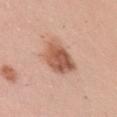biopsy status = total-body-photography surveillance lesion; no biopsy | imaging modality = ~15 mm crop, total-body skin-cancer survey | subject = female, roughly 40 years of age | size = ~4.5 mm (longest diameter) | body site = the right upper arm | automated metrics = a classifier nevus-likeness of about 90/100 and lesion-presence confidence of about 100/100 | tile lighting = white-light illumination.